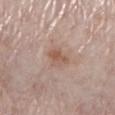* follow-up · imaged on a skin check; not biopsied